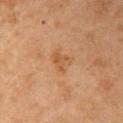<lesion>
<biopsy_status>not biopsied; imaged during a skin examination</biopsy_status>
</lesion>